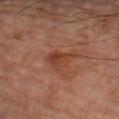This lesion was catalogued during total-body skin photography and was not selected for biopsy.
The lesion is located on the left forearm.
The patient is a male aged around 70.
Imaged with cross-polarized lighting.
The recorded lesion diameter is about 3 mm.
A 15 mm close-up extracted from a 3D total-body photography capture.